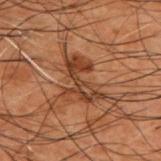Q: Is there a histopathology result?
A: imaged on a skin check; not biopsied
Q: Illumination type?
A: cross-polarized illumination
Q: Patient demographics?
A: male, approximately 50 years of age
Q: Automated lesion metrics?
A: an area of roughly 12 mm², an eccentricity of roughly 0.8, and two-axis asymmetry of about 0.5; an average lesion color of about L≈31 a*≈19 b*≈26 (CIELAB) and a lesion–skin lightness drop of about 8; a border-irregularity index near 8.5/10, internal color variation of about 5.5 on a 0–10 scale, and a peripheral color-asymmetry measure near 2; a classifier nevus-likeness of about 5/100 and a lesion-detection confidence of about 95/100
Q: Lesion size?
A: about 5.5 mm
Q: How was this image acquired?
A: 15 mm crop, total-body photography
Q: Where on the body is the lesion?
A: the upper back